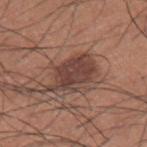This lesion was catalogued during total-body skin photography and was not selected for biopsy. A region of skin cropped from a whole-body photographic capture, roughly 15 mm wide. A male subject in their mid- to late 30s. Longest diameter approximately 5.5 mm. Captured under white-light illumination. On the left upper arm.